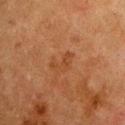  biopsy_status: not biopsied; imaged during a skin examination
  patient:
    sex: female
    age_approx: 50
  image:
    source: total-body photography crop
    field_of_view_mm: 15
  site: chest